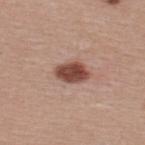This lesion was catalogued during total-body skin photography and was not selected for biopsy. About 4 mm across. A lesion tile, about 15 mm wide, cut from a 3D total-body photograph. The subject is a male aged approximately 40. The tile uses white-light illumination. Located on the back.